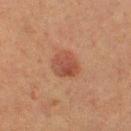Notes:
* notes — catalogued during a skin exam; not biopsied
* image source — total-body-photography crop, ~15 mm field of view
* subject — female, aged around 50
* anatomic site — the left thigh